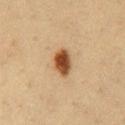Part of a total-body skin-imaging series; this lesion was reviewed on a skin check and was not flagged for biopsy.